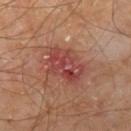{"biopsy_status": "not biopsied; imaged during a skin examination", "lesion_size": {"long_diameter_mm_approx": 4.5}, "image": {"source": "total-body photography crop", "field_of_view_mm": 15}, "automated_metrics": {"border_irregularity_0_10": 2.5, "color_variation_0_10": 7.0, "peripheral_color_asymmetry": 3.0}, "lighting": "cross-polarized", "site": "leg", "patient": {"sex": "male", "age_approx": 60}}